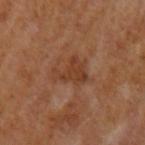Q: Was a biopsy performed?
A: no biopsy performed (imaged during a skin exam)
Q: What lighting was used for the tile?
A: cross-polarized
Q: What are the patient's age and sex?
A: male, in their mid- to late 60s
Q: What is the anatomic site?
A: the left upper arm
Q: What is the lesion's diameter?
A: ≈4 mm
Q: Automated lesion metrics?
A: a lesion color around L≈37 a*≈21 b*≈30 in CIELAB, a lesion–skin lightness drop of about 7, and a lesion-to-skin contrast of about 6.5 (normalized; higher = more distinct); a color-variation rating of about 2/10 and a peripheral color-asymmetry measure near 1; an automated nevus-likeness rating near 0 out of 100 and a detector confidence of about 100 out of 100 that the crop contains a lesion
Q: What is the imaging modality?
A: total-body-photography crop, ~15 mm field of view